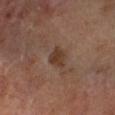follow-up: catalogued during a skin exam; not biopsied | diameter: about 3 mm | anatomic site: the right forearm | patient: male, aged 68 to 72 | lighting: cross-polarized | acquisition: total-body-photography crop, ~15 mm field of view | TBP lesion metrics: an eccentricity of roughly 0.75 and a shape-asymmetry score of about 0.25 (0 = symmetric).